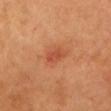  image:
    source: total-body photography crop
    field_of_view_mm: 15
  patient:
    sex: female
    age_approx: 65
  site: head or neck
  lesion_size:
    long_diameter_mm_approx: 3.0
  lighting: cross-polarized